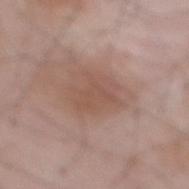Imaged during a routine full-body skin examination; the lesion was not biopsied and no histopathology is available.
A male patient about 65 years old.
A lesion tile, about 15 mm wide, cut from a 3D total-body photograph.
About 4.5 mm across.
Located on the back.
Automated tile analysis of the lesion measured a lesion area of about 10 mm², an eccentricity of roughly 0.65, and two-axis asymmetry of about 0.35. And it measured a mean CIELAB color near L≈52 a*≈19 b*≈26 and a lesion–skin lightness drop of about 7. The software also gave a lesion-detection confidence of about 100/100.